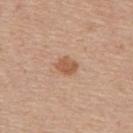Q: Was this lesion biopsied?
A: total-body-photography surveillance lesion; no biopsy
Q: Who is the patient?
A: male, aged 53–57
Q: What kind of image is this?
A: total-body-photography crop, ~15 mm field of view
Q: Lesion location?
A: the back